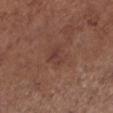From the leg.
Approximately 2.5 mm at its widest.
A female patient aged 53–57.
This is a white-light tile.
A roughly 15 mm field-of-view crop from a total-body skin photograph.